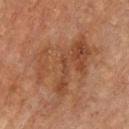Captured during whole-body skin photography for melanoma surveillance; the lesion was not biopsied.
Cropped from a whole-body photographic skin survey; the tile spans about 15 mm.
The tile uses cross-polarized illumination.
The lesion is located on the chest.
A male patient roughly 70 years of age.
The lesion-visualizer software estimated a color-variation rating of about 5/10 and peripheral color asymmetry of about 2. The software also gave a nevus-likeness score of about 10/100 and a detector confidence of about 100 out of 100 that the crop contains a lesion.